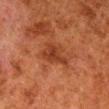The lesion was tiled from a total-body skin photograph and was not biopsied.
Automated tile analysis of the lesion measured roughly 7 lightness units darker than nearby skin and a lesion-to-skin contrast of about 7 (normalized; higher = more distinct). The analysis additionally found a within-lesion color-variation index near 2.5/10 and radial color variation of about 1. The analysis additionally found lesion-presence confidence of about 100/100.
A male patient, aged approximately 80.
A lesion tile, about 15 mm wide, cut from a 3D total-body photograph.
The lesion's longest dimension is about 4 mm.
The tile uses cross-polarized illumination.
From the right lower leg.